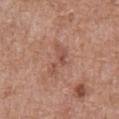Q: Illumination type?
A: white-light illumination
Q: What did automated image analysis measure?
A: an area of roughly 5 mm² and an outline eccentricity of about 0.9 (0 = round, 1 = elongated); a border-irregularity rating of about 7/10, a within-lesion color-variation index near 2/10, and peripheral color asymmetry of about 0.5; a classifier nevus-likeness of about 0/100 and a detector confidence of about 100 out of 100 that the crop contains a lesion
Q: What is the anatomic site?
A: the abdomen
Q: How was this image acquired?
A: total-body-photography crop, ~15 mm field of view
Q: Who is the patient?
A: male, approximately 75 years of age